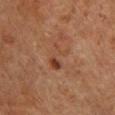workup: catalogued during a skin exam; not biopsied | subject: female, aged 48–52 | TBP lesion metrics: a lesion area of about 5.5 mm², an outline eccentricity of about 0.8 (0 = round, 1 = elongated), and two-axis asymmetry of about 0.45; a lesion-detection confidence of about 100/100 | site: the chest | diameter: ~3.5 mm (longest diameter) | imaging modality: ~15 mm crop, total-body skin-cancer survey | lighting: cross-polarized.